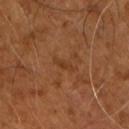Impression:
The lesion was tiled from a total-body skin photograph and was not biopsied.
Image and clinical context:
The patient is a male aged around 65. Longest diameter approximately 3 mm. Automated image analysis of the tile measured a border-irregularity rating of about 3.5/10, internal color variation of about 0 on a 0–10 scale, and peripheral color asymmetry of about 0. This is a cross-polarized tile. A 15 mm close-up extracted from a 3D total-body photography capture.